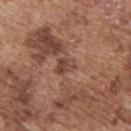anatomic site: the upper back | subject: male, aged around 75 | automated lesion analysis: an area of roughly 3.5 mm², a shape eccentricity near 0.65, and two-axis asymmetry of about 0.4; an average lesion color of about L≈44 a*≈21 b*≈27 (CIELAB), roughly 9 lightness units darker than nearby skin, and a normalized lesion–skin contrast near 7.5 | lesion size: about 2.5 mm | image source: total-body-photography crop, ~15 mm field of view.